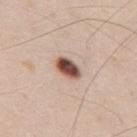Impression: The lesion was tiled from a total-body skin photograph and was not biopsied. Clinical summary: The tile uses white-light illumination. This image is a 15 mm lesion crop taken from a total-body photograph. Automated image analysis of the tile measured a lesion color around L≈51 a*≈20 b*≈25 in CIELAB, roughly 21 lightness units darker than nearby skin, and a lesion-to-skin contrast of about 13.5 (normalized; higher = more distinct). It also reported a nevus-likeness score of about 100/100 and a lesion-detection confidence of about 100/100. Located on the mid back. About 3.5 mm across. A male subject, in their mid- to late 30s.